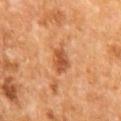Q: Was a biopsy performed?
A: catalogued during a skin exam; not biopsied
Q: What is the imaging modality?
A: ~15 mm tile from a whole-body skin photo
Q: Where on the body is the lesion?
A: the mid back
Q: Illumination type?
A: cross-polarized illumination
Q: Who is the patient?
A: male, in their mid-60s
Q: Automated lesion metrics?
A: a border-irregularity rating of about 3/10, a color-variation rating of about 3.5/10, and radial color variation of about 1
Q: How large is the lesion?
A: ~4 mm (longest diameter)